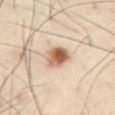  biopsy_status: not biopsied; imaged during a skin examination
  lighting: cross-polarized
  site: front of the torso
  image:
    source: total-body photography crop
    field_of_view_mm: 15
  patient:
    sex: male
    age_approx: 55
  automated_metrics:
    area_mm2_approx: 7.5
    eccentricity: 0.55
    shape_asymmetry: 0.15
    nevus_likeness_0_100: 100
  lesion_size:
    long_diameter_mm_approx: 3.5